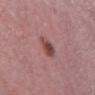Assessment:
The lesion was tiled from a total-body skin photograph and was not biopsied.
Clinical summary:
The lesion is located on the right lower leg. This is a white-light tile. The patient is a female aged 38–42. A close-up tile cropped from a whole-body skin photograph, about 15 mm across. An algorithmic analysis of the crop reported a footprint of about 5.5 mm², an outline eccentricity of about 0.45 (0 = round, 1 = elongated), and a shape-asymmetry score of about 0.15 (0 = symmetric). The software also gave a border-irregularity index near 1.5/10, internal color variation of about 5 on a 0–10 scale, and a peripheral color-asymmetry measure near 1.5. The recorded lesion diameter is about 2.5 mm.